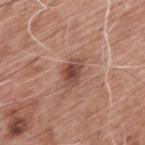Impression:
Captured during whole-body skin photography for melanoma surveillance; the lesion was not biopsied.
Clinical summary:
On the upper back. This is a white-light tile. Automated image analysis of the tile measured a border-irregularity index near 3/10, internal color variation of about 4 on a 0–10 scale, and a peripheral color-asymmetry measure near 1. The software also gave an automated nevus-likeness rating near 70 out of 100 and a lesion-detection confidence of about 100/100. A 15 mm crop from a total-body photograph taken for skin-cancer surveillance. The subject is a male aged approximately 60.